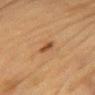{
  "biopsy_status": "not biopsied; imaged during a skin examination",
  "site": "chest",
  "lesion_size": {
    "long_diameter_mm_approx": 2.5
  },
  "patient": {
    "sex": "female",
    "age_approx": 80
  },
  "image": {
    "source": "total-body photography crop",
    "field_of_view_mm": 15
  },
  "lighting": "cross-polarized"
}